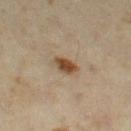<tbp_lesion>
<biopsy_status>not biopsied; imaged during a skin examination</biopsy_status>
<automated_metrics>
  <cielab_L>45</cielab_L>
  <cielab_a>16</cielab_a>
  <cielab_b>32</cielab_b>
  <vs_skin_contrast_norm>10.5</vs_skin_contrast_norm>
  <nevus_likeness_0_100>100</nevus_likeness_0_100>
  <lesion_detection_confidence_0_100>100</lesion_detection_confidence_0_100>
</automated_metrics>
<lighting>cross-polarized</lighting>
<patient>
  <sex>female</sex>
  <age_approx>35</age_approx>
</patient>
<image>
  <source>total-body photography crop</source>
  <field_of_view_mm>15</field_of_view_mm>
</image>
<site>leg</site>
</tbp_lesion>